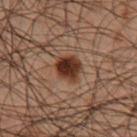The lesion was tiled from a total-body skin photograph and was not biopsied. The total-body-photography lesion software estimated a mean CIELAB color near L≈24 a*≈17 b*≈22 and a lesion-to-skin contrast of about 13.5 (normalized; higher = more distinct). The software also gave a border-irregularity rating of about 2/10 and peripheral color asymmetry of about 1.5. The subject is a male approximately 50 years of age. The lesion's longest dimension is about 3.5 mm. On the right lower leg. The tile uses cross-polarized illumination. A 15 mm close-up tile from a total-body photography series done for melanoma screening.